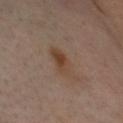The lesion was photographed on a routine skin check and not biopsied; there is no pathology result. The lesion's longest dimension is about 4 mm. The total-body-photography lesion software estimated an average lesion color of about L≈42 a*≈16 b*≈28 (CIELAB) and about 7 CIELAB-L* units darker than the surrounding skin. And it measured a nevus-likeness score of about 60/100 and a detector confidence of about 100 out of 100 that the crop contains a lesion. From the head or neck. The tile uses cross-polarized illumination. A male patient, about 55 years old. A roughly 15 mm field-of-view crop from a total-body skin photograph.